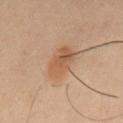| feature | finding |
|---|---|
| follow-up | imaged on a skin check; not biopsied |
| acquisition | 15 mm crop, total-body photography |
| TBP lesion metrics | a border-irregularity index near 2/10 and radial color variation of about 1.5; a classifier nevus-likeness of about 80/100 and a detector confidence of about 100 out of 100 that the crop contains a lesion |
| subject | male, roughly 40 years of age |
| size | about 4.5 mm |
| anatomic site | the mid back |
| tile lighting | cross-polarized |